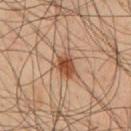Acquisition and patient details:
The total-body-photography lesion software estimated a shape eccentricity near 0.7 and a shape-asymmetry score of about 0.3 (0 = symmetric). It also reported a mean CIELAB color near L≈47 a*≈22 b*≈32, about 12 CIELAB-L* units darker than the surrounding skin, and a normalized border contrast of about 9.5. The analysis additionally found a within-lesion color-variation index near 4/10 and a peripheral color-asymmetry measure near 1.5. The analysis additionally found an automated nevus-likeness rating near 95 out of 100 and a lesion-detection confidence of about 100/100. The patient is a male about 45 years old. The lesion is located on the chest. Imaged with cross-polarized lighting. The lesion's longest dimension is about 3 mm. A 15 mm close-up tile from a total-body photography series done for melanoma screening.